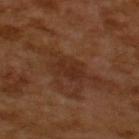Imaged during a routine full-body skin examination; the lesion was not biopsied and no histopathology is available.